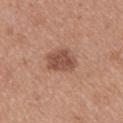| key | value |
|---|---|
| notes | total-body-photography surveillance lesion; no biopsy |
| tile lighting | white-light illumination |
| diameter | ≈3.5 mm |
| automated lesion analysis | a border-irregularity rating of about 3/10 and internal color variation of about 2.5 on a 0–10 scale |
| image source | ~15 mm tile from a whole-body skin photo |
| anatomic site | the upper back |
| subject | female, aged 33–37 |